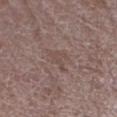Q: Was this lesion biopsied?
A: imaged on a skin check; not biopsied
Q: What is the anatomic site?
A: the right upper arm
Q: What is the imaging modality?
A: ~15 mm crop, total-body skin-cancer survey
Q: What lighting was used for the tile?
A: white-light
Q: What are the patient's age and sex?
A: female, approximately 70 years of age
Q: Lesion size?
A: ≈3 mm